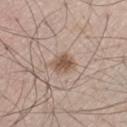Assessment:
Recorded during total-body skin imaging; not selected for excision or biopsy.
Context:
A male subject in their mid- to late 40s. From the leg. Automated tile analysis of the lesion measured a footprint of about 5.5 mm², an eccentricity of roughly 0.7, and two-axis asymmetry of about 0.15. It also reported an average lesion color of about L≈54 a*≈16 b*≈27 (CIELAB). About 3 mm across. Imaged with white-light lighting. Cropped from a whole-body photographic skin survey; the tile spans about 15 mm.